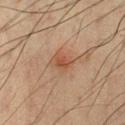{
  "biopsy_status": "not biopsied; imaged during a skin examination",
  "lesion_size": {
    "long_diameter_mm_approx": 3.0
  },
  "patient": {
    "sex": "male",
    "age_approx": 60
  },
  "site": "chest",
  "image": {
    "source": "total-body photography crop",
    "field_of_view_mm": 15
  },
  "automated_metrics": {
    "border_irregularity_0_10": 2.0,
    "color_variation_0_10": 3.0,
    "peripheral_color_asymmetry": 1.0
  }
}